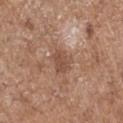The lesion was tiled from a total-body skin photograph and was not biopsied.
This image is a 15 mm lesion crop taken from a total-body photograph.
A male patient, approximately 70 years of age.
The recorded lesion diameter is about 3 mm.
The lesion is located on the left upper arm.
Captured under white-light illumination.
Automated image analysis of the tile measured a nevus-likeness score of about 0/100 and lesion-presence confidence of about 100/100.